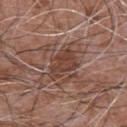workup — imaged on a skin check; not biopsied
tile lighting — white-light
image source — ~15 mm tile from a whole-body skin photo
location — the front of the torso
diameter — ≈4.5 mm
patient — male, aged approximately 75